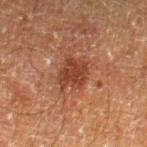  biopsy_status: not biopsied; imaged during a skin examination
  lesion_size:
    long_diameter_mm_approx: 4.0
  patient:
    sex: male
    age_approx: 60
  automated_metrics:
    nevus_likeness_0_100: 35
  image:
    source: total-body photography crop
    field_of_view_mm: 15
  site: leg